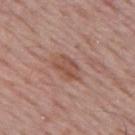Clinical impression:
Part of a total-body skin-imaging series; this lesion was reviewed on a skin check and was not flagged for biopsy.
Acquisition and patient details:
On the back. Cropped from a whole-body photographic skin survey; the tile spans about 15 mm. Automated tile analysis of the lesion measured a lesion area of about 7 mm², a shape eccentricity near 0.8, and a shape-asymmetry score of about 0.3 (0 = symmetric). And it measured an average lesion color of about L≈51 a*≈21 b*≈27 (CIELAB), a lesion–skin lightness drop of about 8, and a normalized lesion–skin contrast near 6.5. It also reported a border-irregularity rating of about 3/10, a color-variation rating of about 3.5/10, and peripheral color asymmetry of about 1. And it measured a nevus-likeness score of about 0/100 and lesion-presence confidence of about 100/100. A male patient aged approximately 65. Approximately 4 mm at its widest. Imaged with white-light lighting.